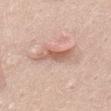Notes:
- workup — imaged on a skin check; not biopsied
- imaging modality — ~15 mm tile from a whole-body skin photo
- location — the upper back
- illumination — white-light
- automated lesion analysis — a footprint of about 9 mm² and two-axis asymmetry of about 0.35; a within-lesion color-variation index near 4.5/10 and a peripheral color-asymmetry measure near 1.5; a classifier nevus-likeness of about 60/100
- subject — male, roughly 50 years of age